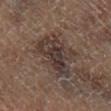No biopsy was performed on this lesion — it was imaged during a full skin examination and was not determined to be concerning. The subject is a male approximately 65 years of age. The lesion-visualizer software estimated a footprint of about 21 mm², an outline eccentricity of about 0.65 (0 = round, 1 = elongated), and two-axis asymmetry of about 0.35. The software also gave a mean CIELAB color near L≈33 a*≈12 b*≈18 and a lesion-to-skin contrast of about 8.5 (normalized; higher = more distinct). On the right lower leg. Approximately 6 mm at its widest. A lesion tile, about 15 mm wide, cut from a 3D total-body photograph.